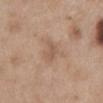follow-up=imaged on a skin check; not biopsied | anatomic site=the abdomen | image=15 mm crop, total-body photography | patient=female, aged around 40 | diameter=~3.5 mm (longest diameter).